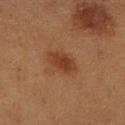site: leg
lighting: cross-polarized
image:
  source: total-body photography crop
  field_of_view_mm: 15
patient:
  sex: female
  age_approx: 40